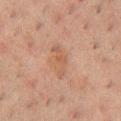Recorded during total-body skin imaging; not selected for excision or biopsy. A close-up tile cropped from a whole-body skin photograph, about 15 mm across. From the front of the torso. Captured under cross-polarized illumination. The total-body-photography lesion software estimated an eccentricity of roughly 0.9 and a symmetry-axis asymmetry near 0.5. The analysis additionally found a classifier nevus-likeness of about 0/100 and a detector confidence of about 100 out of 100 that the crop contains a lesion. A male patient roughly 50 years of age. The lesion's longest dimension is about 4 mm.